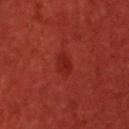Notes:
* follow-up: catalogued during a skin exam; not biopsied
* imaging modality: ~15 mm tile from a whole-body skin photo
* illumination: cross-polarized
* diameter: about 3 mm
* image-analysis metrics: a lesion area of about 4.5 mm², an eccentricity of roughly 0.85, and a shape-asymmetry score of about 0.2 (0 = symmetric); about 5 CIELAB-L* units darker than the surrounding skin and a normalized lesion–skin contrast near 5
* anatomic site: the head or neck
* patient: male, roughly 60 years of age